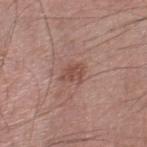Q: Is there a histopathology result?
A: total-body-photography surveillance lesion; no biopsy
Q: What is the imaging modality?
A: total-body-photography crop, ~15 mm field of view
Q: Who is the patient?
A: male, aged around 60
Q: Where on the body is the lesion?
A: the right lower leg
Q: Illumination type?
A: white-light
Q: How large is the lesion?
A: ≈2.5 mm